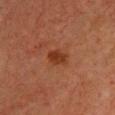workup=catalogued during a skin exam; not biopsied | illumination=cross-polarized illumination | patient=female, aged around 40 | image source=total-body-photography crop, ~15 mm field of view | size=~2.5 mm (longest diameter) | body site=the front of the torso.